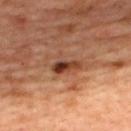Q: Was this lesion biopsied?
A: imaged on a skin check; not biopsied